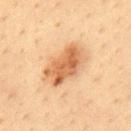• workup: total-body-photography surveillance lesion; no biopsy
• lighting: cross-polarized illumination
• automated lesion analysis: a lesion color around L≈60 a*≈22 b*≈38 in CIELAB, a lesion–skin lightness drop of about 13, and a normalized lesion–skin contrast near 8; radial color variation of about 2; a classifier nevus-likeness of about 90/100 and lesion-presence confidence of about 100/100
• diameter: ≈6 mm
• site: the upper back
• image: total-body-photography crop, ~15 mm field of view
• patient: male, about 35 years old